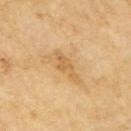lesion_size:
  long_diameter_mm_approx: 4.0
automated_metrics:
  cielab_L: 64
  cielab_a: 19
  cielab_b: 44
  vs_skin_darker_L: 8.0
  vs_skin_contrast_norm: 6.0
site: right upper arm
image:
  source: total-body photography crop
  field_of_view_mm: 15
patient:
  sex: female
  age_approx: 70